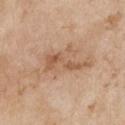Assessment: Recorded during total-body skin imaging; not selected for excision or biopsy.